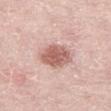– notes: imaged on a skin check; not biopsied
– image source: ~15 mm tile from a whole-body skin photo
– lighting: white-light illumination
– site: the right thigh
– size: about 5 mm
– subject: male, aged 48–52
– TBP lesion metrics: a border-irregularity rating of about 1.5/10 and internal color variation of about 4 on a 0–10 scale